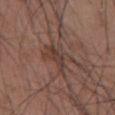The lesion was photographed on a routine skin check and not biopsied; there is no pathology result.
About 4 mm across.
A male patient, in their mid-50s.
The lesion is located on the front of the torso.
Cropped from a total-body skin-imaging series; the visible field is about 15 mm.
The lesion-visualizer software estimated a footprint of about 8 mm², an outline eccentricity of about 0.8 (0 = round, 1 = elongated), and a shape-asymmetry score of about 0.35 (0 = symmetric). And it measured a color-variation rating of about 4/10. The analysis additionally found an automated nevus-likeness rating near 15 out of 100 and a detector confidence of about 75 out of 100 that the crop contains a lesion.
Imaged with white-light lighting.